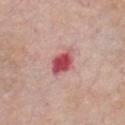Q: Where on the body is the lesion?
A: the chest
Q: How was this image acquired?
A: ~15 mm tile from a whole-body skin photo
Q: Illumination type?
A: white-light
Q: Automated lesion metrics?
A: a nevus-likeness score of about 0/100 and a lesion-detection confidence of about 100/100
Q: What are the patient's age and sex?
A: male, aged approximately 65